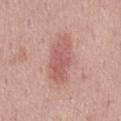Case summary:
* biopsy status: catalogued during a skin exam; not biopsied
* location: the mid back
* imaging modality: ~15 mm tile from a whole-body skin photo
* lesion size: ≈6 mm
* lighting: white-light illumination
* subject: male, in their mid-40s